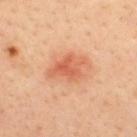This lesion was catalogued during total-body skin photography and was not selected for biopsy.
From the upper back.
A close-up tile cropped from a whole-body skin photograph, about 15 mm across.
A male patient roughly 35 years of age.
Longest diameter approximately 5 mm.
Imaged with cross-polarized lighting.